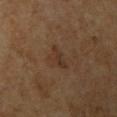Impression:
Recorded during total-body skin imaging; not selected for excision or biopsy.
Acquisition and patient details:
Cropped from a whole-body photographic skin survey; the tile spans about 15 mm. The lesion is on the right upper arm. The patient is a male roughly 60 years of age. This is a cross-polarized tile.